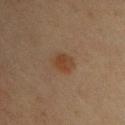biopsy_status: not biopsied; imaged during a skin examination
patient:
  sex: female
  age_approx: 65
lighting: cross-polarized
image:
  source: total-body photography crop
  field_of_view_mm: 15
site: chest
automated_metrics:
  area_mm2_approx: 5.0
  eccentricity: 0.6
  shape_asymmetry: 0.2
  border_irregularity_0_10: 2.0
  color_variation_0_10: 3.0
  peripheral_color_asymmetry: 1.0
  nevus_likeness_0_100: 90
  lesion_detection_confidence_0_100: 100